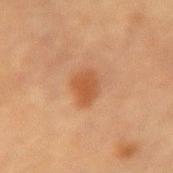Context: On the right forearm. A female patient, aged 53–57. This image is a 15 mm lesion crop taken from a total-body photograph.